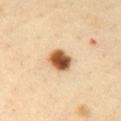Clinical impression:
Recorded during total-body skin imaging; not selected for excision or biopsy.
Context:
A 15 mm crop from a total-body photograph taken for skin-cancer surveillance. On the mid back. Imaged with cross-polarized lighting. A female patient, aged 43–47. The lesion-visualizer software estimated a shape eccentricity near 0.5.